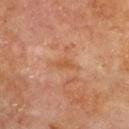The lesion was photographed on a routine skin check and not biopsied; there is no pathology result. Captured under cross-polarized illumination. The recorded lesion diameter is about 2.5 mm. The lesion is located on the upper back. Automated tile analysis of the lesion measured an area of roughly 2.5 mm², a shape eccentricity near 0.9, and two-axis asymmetry of about 0.35. The software also gave an average lesion color of about L≈46 a*≈22 b*≈33 (CIELAB) and about 6 CIELAB-L* units darker than the surrounding skin. The analysis additionally found a border-irregularity index near 3.5/10, a within-lesion color-variation index near 0/10, and radial color variation of about 0. The software also gave an automated nevus-likeness rating near 0 out of 100 and a detector confidence of about 100 out of 100 that the crop contains a lesion. A close-up tile cropped from a whole-body skin photograph, about 15 mm across. The patient is a female aged 78 to 82.